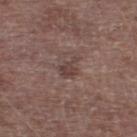A 15 mm crop from a total-body photograph taken for skin-cancer surveillance. A male patient, in their 70s. The lesion is on the left thigh. The tile uses white-light illumination.